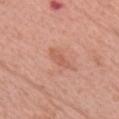Part of a total-body skin-imaging series; this lesion was reviewed on a skin check and was not flagged for biopsy.
A female patient, about 60 years old.
About 3.5 mm across.
The lesion is located on the chest.
The tile uses white-light illumination.
Cropped from a whole-body photographic skin survey; the tile spans about 15 mm.
Automated tile analysis of the lesion measured a footprint of about 3.5 mm², an eccentricity of roughly 0.9, and a shape-asymmetry score of about 0.25 (0 = symmetric). The software also gave a nevus-likeness score of about 5/100 and lesion-presence confidence of about 100/100.